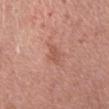Case summary:
– notes · catalogued during a skin exam; not biopsied
– lesion diameter · ≈3 mm
– body site · the arm
– image source · ~15 mm crop, total-body skin-cancer survey
– lighting · white-light illumination
– patient · female, in their mid- to late 30s
– automated metrics · a border-irregularity rating of about 3.5/10 and radial color variation of about 0.5; a classifier nevus-likeness of about 0/100 and a detector confidence of about 100 out of 100 that the crop contains a lesion